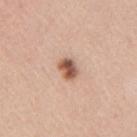biopsy status — no biopsy performed (imaged during a skin exam)
acquisition — 15 mm crop, total-body photography
anatomic site — the right upper arm
tile lighting — white-light
diameter — ~2.5 mm (longest diameter)
subject — female, aged 38 to 42
image-analysis metrics — an average lesion color of about L≈55 a*≈22 b*≈29 (CIELAB), about 17 CIELAB-L* units darker than the surrounding skin, and a normalized lesion–skin contrast near 10.5; lesion-presence confidence of about 100/100